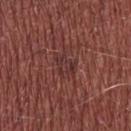– workup · imaged on a skin check; not biopsied
– patient · male, aged 53–57
– image · total-body-photography crop, ~15 mm field of view
– site · the upper back
– diameter · ≈3 mm